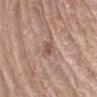follow-up = imaged on a skin check; not biopsied
lesion size = ≈3 mm
tile lighting = white-light
anatomic site = the right forearm
imaging modality = 15 mm crop, total-body photography
patient = female, approximately 75 years of age
image-analysis metrics = a nevus-likeness score of about 0/100 and lesion-presence confidence of about 100/100